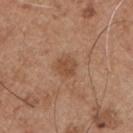The lesion was photographed on a routine skin check and not biopsied; there is no pathology result. An algorithmic analysis of the crop reported a lesion area of about 4.5 mm², an eccentricity of roughly 0.5, and a shape-asymmetry score of about 0.25 (0 = symmetric). It also reported a lesion–skin lightness drop of about 8 and a normalized lesion–skin contrast near 6. The software also gave a classifier nevus-likeness of about 20/100. The lesion is on the chest. Captured under white-light illumination. This image is a 15 mm lesion crop taken from a total-body photograph. About 2.5 mm across. A male subject aged approximately 55.